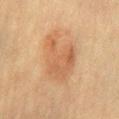Imaged during a routine full-body skin examination; the lesion was not biopsied and no histopathology is available. A lesion tile, about 15 mm wide, cut from a 3D total-body photograph. The lesion is on the mid back. A male patient, approximately 60 years of age. Captured under cross-polarized illumination. The lesion-visualizer software estimated a lesion–skin lightness drop of about 8 and a normalized border contrast of about 6. It also reported a border-irregularity index near 4.5/10 and a within-lesion color-variation index near 4/10.